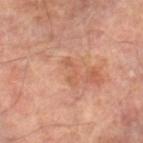Image and clinical context:
The lesion is located on the right thigh. A male subject, about 65 years old. Cropped from a total-body skin-imaging series; the visible field is about 15 mm.